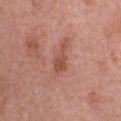No biopsy was performed on this lesion — it was imaged during a full skin examination and was not determined to be concerning. A 15 mm close-up tile from a total-body photography series done for melanoma screening. On the back. A female patient in their mid- to late 60s.